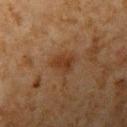No biopsy was performed on this lesion — it was imaged during a full skin examination and was not determined to be concerning. On the left upper arm. This image is a 15 mm lesion crop taken from a total-body photograph. A male subject aged 58 to 62.